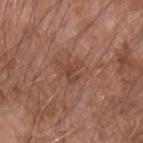<tbp_lesion>
  <biopsy_status>not biopsied; imaged during a skin examination</biopsy_status>
  <site>left forearm</site>
  <lesion_size>
    <long_diameter_mm_approx>3.0</long_diameter_mm_approx>
  </lesion_size>
  <image>
    <source>total-body photography crop</source>
    <field_of_view_mm>15</field_of_view_mm>
  </image>
  <lighting>white-light</lighting>
  <patient>
    <sex>male</sex>
    <age_approx>60</age_approx>
  </patient>
</tbp_lesion>